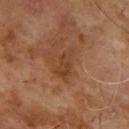| feature | finding |
|---|---|
| workup | total-body-photography surveillance lesion; no biopsy |
| imaging modality | total-body-photography crop, ~15 mm field of view |
| patient | male, aged around 65 |
| location | the back |
| size | about 3.5 mm |
| lighting | cross-polarized |